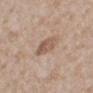Q: Was this lesion biopsied?
A: catalogued during a skin exam; not biopsied
Q: How was this image acquired?
A: total-body-photography crop, ~15 mm field of view
Q: What are the patient's age and sex?
A: male, in their mid-60s
Q: What is the lesion's diameter?
A: ~3.5 mm (longest diameter)
Q: Lesion location?
A: the mid back
Q: Illumination type?
A: white-light illumination
Q: Automated lesion metrics?
A: a shape eccentricity near 0.75